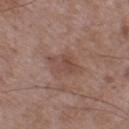<tbp_lesion>
<biopsy_status>not biopsied; imaged during a skin examination</biopsy_status>
<image>
  <source>total-body photography crop</source>
  <field_of_view_mm>15</field_of_view_mm>
</image>
<patient>
  <sex>male</sex>
  <age_approx>50</age_approx>
</patient>
<site>left thigh</site>
<automated_metrics>
  <area_mm2_approx>6.0</area_mm2_approx>
  <shape_asymmetry>0.25</shape_asymmetry>
  <cielab_L>46</cielab_L>
  <cielab_a>19</cielab_a>
  <cielab_b>24</cielab_b>
  <border_irregularity_0_10>3.5</border_irregularity_0_10>
  <color_variation_0_10>3.0</color_variation_0_10>
  <peripheral_color_asymmetry>1.0</peripheral_color_asymmetry>
</automated_metrics>
<lighting>white-light</lighting>
</tbp_lesion>